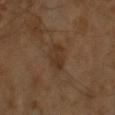Imaged during a routine full-body skin examination; the lesion was not biopsied and no histopathology is available.
Cropped from a whole-body photographic skin survey; the tile spans about 15 mm.
An algorithmic analysis of the crop reported a lesion color around L≈32 a*≈16 b*≈27 in CIELAB, roughly 6 lightness units darker than nearby skin, and a normalized border contrast of about 6.5. The analysis additionally found border irregularity of about 2 on a 0–10 scale.
The tile uses cross-polarized illumination.
A male subject aged 63–67.